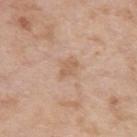This lesion was catalogued during total-body skin photography and was not selected for biopsy. A male subject, aged around 60. A close-up tile cropped from a whole-body skin photograph, about 15 mm across. On the left upper arm. Approximately 2.5 mm at its widest. Captured under white-light illumination.